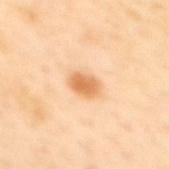follow-up = no biopsy performed (imaged during a skin exam) | lesion size = about 3.5 mm | acquisition = ~15 mm crop, total-body skin-cancer survey | automated metrics = a peripheral color-asymmetry measure near 1; a lesion-detection confidence of about 100/100 | anatomic site = the upper back | subject = female, in their mid- to late 40s.